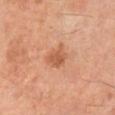<record>
<biopsy_status>not biopsied; imaged during a skin examination</biopsy_status>
<automated_metrics>
  <area_mm2_approx>6.0</area_mm2_approx>
  <eccentricity>0.5</eccentricity>
  <shape_asymmetry>0.3</shape_asymmetry>
  <cielab_L>53</cielab_L>
  <cielab_a>24</cielab_a>
  <cielab_b>33</cielab_b>
  <vs_skin_darker_L>9.0</vs_skin_darker_L>
  <vs_skin_contrast_norm>6.5</vs_skin_contrast_norm>
</automated_metrics>
<lighting>cross-polarized</lighting>
<patient>
  <sex>male</sex>
  <age_approx>60</age_approx>
</patient>
<image>
  <source>total-body photography crop</source>
  <field_of_view_mm>15</field_of_view_mm>
</image>
<site>right lower leg</site>
<lesion_size>
  <long_diameter_mm_approx>3.0</long_diameter_mm_approx>
</lesion_size>
</record>